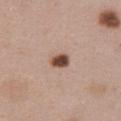Part of a total-body skin-imaging series; this lesion was reviewed on a skin check and was not flagged for biopsy.
The tile uses white-light illumination.
Located on the abdomen.
The lesion-visualizer software estimated a lesion area of about 4 mm², a shape eccentricity near 0.7, and two-axis asymmetry of about 0.15. And it measured a lesion color around L≈48 a*≈20 b*≈26 in CIELAB, roughly 18 lightness units darker than nearby skin, and a normalized lesion–skin contrast near 12.5. And it measured an automated nevus-likeness rating near 100 out of 100 and lesion-presence confidence of about 100/100.
A close-up tile cropped from a whole-body skin photograph, about 15 mm across.
The patient is a female in their 40s.